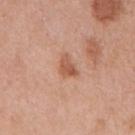Part of a total-body skin-imaging series; this lesion was reviewed on a skin check and was not flagged for biopsy. The patient is a male aged approximately 70. Longest diameter approximately 3 mm. A lesion tile, about 15 mm wide, cut from a 3D total-body photograph. Located on the mid back. The tile uses white-light illumination.